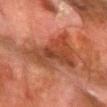  biopsy_status: not biopsied; imaged during a skin examination
  image:
    source: total-body photography crop
    field_of_view_mm: 15
  lighting: cross-polarized
  site: left forearm
  patient:
    sex: male
    age_approx: 80